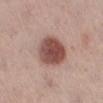Q: Was this lesion biopsied?
A: catalogued during a skin exam; not biopsied
Q: What kind of image is this?
A: ~15 mm crop, total-body skin-cancer survey
Q: Lesion size?
A: ≈4.5 mm
Q: Patient demographics?
A: female, roughly 40 years of age
Q: Automated lesion metrics?
A: a mean CIELAB color near L≈49 a*≈22 b*≈23 and a lesion–skin lightness drop of about 16; a border-irregularity rating of about 1.5/10 and radial color variation of about 1.5
Q: What is the anatomic site?
A: the right lower leg
Q: Illumination type?
A: white-light